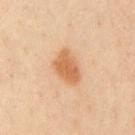The lesion was tiled from a total-body skin photograph and was not biopsied. Measured at roughly 4 mm in maximum diameter. The total-body-photography lesion software estimated a lesion color around L≈64 a*≈23 b*≈40 in CIELAB, roughly 12 lightness units darker than nearby skin, and a lesion-to-skin contrast of about 8 (normalized; higher = more distinct). And it measured a border-irregularity rating of about 2/10, a color-variation rating of about 3/10, and peripheral color asymmetry of about 1. It also reported a nevus-likeness score of about 95/100. A 15 mm close-up extracted from a 3D total-body photography capture. The tile uses cross-polarized illumination. The lesion is on the front of the torso. A male patient aged 38 to 42.